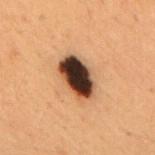{
  "biopsy_status": "not biopsied; imaged during a skin examination",
  "lighting": "cross-polarized",
  "image": {
    "source": "total-body photography crop",
    "field_of_view_mm": 15
  },
  "site": "upper back",
  "patient": {
    "sex": "male",
    "age_approx": 50
  },
  "automated_metrics": {
    "color_variation_0_10": 10.0,
    "peripheral_color_asymmetry": 3.0
  },
  "lesion_size": {
    "long_diameter_mm_approx": 5.0
  }
}